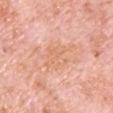Q: Was this lesion biopsied?
A: catalogued during a skin exam; not biopsied
Q: Illumination type?
A: white-light
Q: What is the lesion's diameter?
A: about 5.5 mm
Q: How was this image acquired?
A: 15 mm crop, total-body photography
Q: What did automated image analysis measure?
A: a lesion–skin lightness drop of about 6
Q: What is the anatomic site?
A: the chest
Q: What are the patient's age and sex?
A: male, about 80 years old